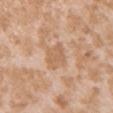| field | value |
|---|---|
| workup | no biopsy performed (imaged during a skin exam) |
| TBP lesion metrics | an average lesion color of about L≈62 a*≈19 b*≈34 (CIELAB), a lesion–skin lightness drop of about 8, and a normalized border contrast of about 5.5; border irregularity of about 3.5 on a 0–10 scale, internal color variation of about 1.5 on a 0–10 scale, and peripheral color asymmetry of about 0.5; a nevus-likeness score of about 0/100 |
| image | 15 mm crop, total-body photography |
| body site | the chest |
| subject | female, approximately 25 years of age |
| lighting | white-light illumination |